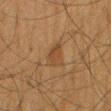This lesion was catalogued during total-body skin photography and was not selected for biopsy. A close-up tile cropped from a whole-body skin photograph, about 15 mm across. Longest diameter approximately 3 mm. Located on the mid back. A male subject in their mid- to late 60s.